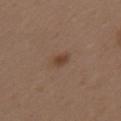| feature | finding |
|---|---|
| follow-up | no biopsy performed (imaged during a skin exam) |
| illumination | white-light illumination |
| image source | ~15 mm tile from a whole-body skin photo |
| subject | female, approximately 40 years of age |
| diameter | ~2.5 mm (longest diameter) |
| body site | the left upper arm |